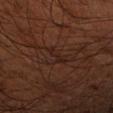| feature | finding |
|---|---|
| subject | male, in their 50s |
| imaging modality | ~15 mm tile from a whole-body skin photo |
| anatomic site | the arm |
| lesion size | ≈2.5 mm |